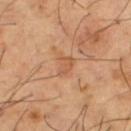Clinical impression:
This lesion was catalogued during total-body skin photography and was not selected for biopsy.
Clinical summary:
A male patient roughly 65 years of age. A region of skin cropped from a whole-body photographic capture, roughly 15 mm wide. On the left thigh.